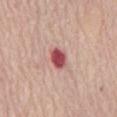Findings:
* workup · catalogued during a skin exam; not biopsied
* TBP lesion metrics · a footprint of about 4.5 mm², an outline eccentricity of about 0.65 (0 = round, 1 = elongated), and a shape-asymmetry score of about 0.2 (0 = symmetric); a border-irregularity rating of about 2/10, a within-lesion color-variation index near 3/10, and peripheral color asymmetry of about 1; a classifier nevus-likeness of about 0/100 and lesion-presence confidence of about 100/100
* lesion size · about 2.5 mm
* anatomic site · the mid back
* tile lighting · white-light
* acquisition · ~15 mm crop, total-body skin-cancer survey
* patient · female, aged around 65